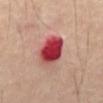Q: Is there a histopathology result?
A: imaged on a skin check; not biopsied
Q: How was this image acquired?
A: ~15 mm crop, total-body skin-cancer survey
Q: Lesion location?
A: the front of the torso
Q: Automated lesion metrics?
A: an average lesion color of about L≈42 a*≈38 b*≈24 (CIELAB) and a lesion-to-skin contrast of about 13.5 (normalized; higher = more distinct)
Q: What is the lesion's diameter?
A: ~4 mm (longest diameter)
Q: What are the patient's age and sex?
A: male, aged approximately 45
Q: How was the tile lit?
A: cross-polarized